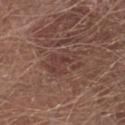This lesion was catalogued during total-body skin photography and was not selected for biopsy. A male subject, approximately 65 years of age. A lesion tile, about 15 mm wide, cut from a 3D total-body photograph. The lesion's longest dimension is about 5 mm. Captured under white-light illumination. Located on the right lower leg.